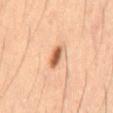The lesion was tiled from a total-body skin photograph and was not biopsied. This image is a 15 mm lesion crop taken from a total-body photograph. The lesion is on the abdomen. A male subject, aged 53 to 57.